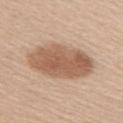follow-up — catalogued during a skin exam; not biopsied
body site — the right upper arm
image source — ~15 mm crop, total-body skin-cancer survey
lighting — white-light
subject — female, aged 38–42
diameter — about 7.5 mm